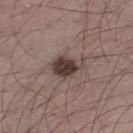follow-up = total-body-photography surveillance lesion; no biopsy
lighting = white-light illumination
image source = ~15 mm crop, total-body skin-cancer survey
diameter = ~4 mm (longest diameter)
location = the left thigh
subject = male, in their mid- to late 30s